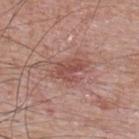Clinical impression: No biopsy was performed on this lesion — it was imaged during a full skin examination and was not determined to be concerning. Background: A close-up tile cropped from a whole-body skin photograph, about 15 mm across. The lesion's longest dimension is about 4.5 mm. A male patient, aged 63 to 67. The lesion is located on the upper back.